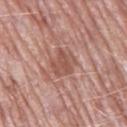Q: Is there a histopathology result?
A: total-body-photography surveillance lesion; no biopsy
Q: How large is the lesion?
A: about 3.5 mm
Q: Where on the body is the lesion?
A: the left thigh
Q: How was this image acquired?
A: ~15 mm tile from a whole-body skin photo
Q: How was the tile lit?
A: white-light
Q: Patient demographics?
A: female, aged approximately 70